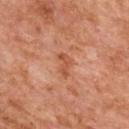Approximately 3 mm at its widest. A 15 mm crop from a total-body photograph taken for skin-cancer surveillance. The lesion-visualizer software estimated a footprint of about 3 mm², an outline eccentricity of about 0.9 (0 = round, 1 = elongated), and a symmetry-axis asymmetry near 0.45. It also reported a lesion color around L≈45 a*≈24 b*≈31 in CIELAB, roughly 8 lightness units darker than nearby skin, and a lesion-to-skin contrast of about 6.5 (normalized; higher = more distinct). And it measured internal color variation of about 0 on a 0–10 scale and a peripheral color-asymmetry measure near 0. And it measured an automated nevus-likeness rating near 0 out of 100 and a lesion-detection confidence of about 100/100. A female subject aged 58–62. Captured under cross-polarized illumination. The lesion is located on the back.